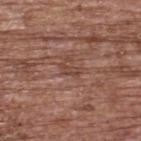Recorded during total-body skin imaging; not selected for excision or biopsy. A female subject in their mid-60s. This image is a 15 mm lesion crop taken from a total-body photograph. Located on the upper back.